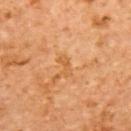image:
  source: total-body photography crop
  field_of_view_mm: 15
patient:
  sex: female
  age_approx: 55
site: upper back